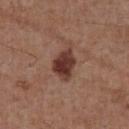workup=no biopsy performed (imaged during a skin exam); patient=male, in their mid- to late 50s; tile lighting=white-light; imaging modality=~15 mm tile from a whole-body skin photo; site=the front of the torso.